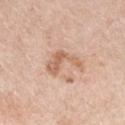A 15 mm close-up extracted from a 3D total-body photography capture.
Imaged with white-light lighting.
Measured at roughly 4 mm in maximum diameter.
On the chest.
The total-body-photography lesion software estimated a lesion color around L≈63 a*≈20 b*≈32 in CIELAB and a normalized lesion–skin contrast near 7. And it measured a nevus-likeness score of about 20/100 and a lesion-detection confidence of about 100/100.
A male patient aged approximately 50.